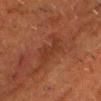<case>
<biopsy_status>not biopsied; imaged during a skin examination</biopsy_status>
<patient>
  <sex>male</sex>
  <age_approx>75</age_approx>
</patient>
<lighting>cross-polarized</lighting>
<site>head or neck</site>
<lesion_size>
  <long_diameter_mm_approx>4.0</long_diameter_mm_approx>
</lesion_size>
<image>
  <source>total-body photography crop</source>
  <field_of_view_mm>15</field_of_view_mm>
</image>
</case>